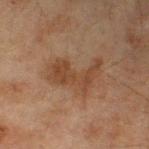| field | value |
|---|---|
| notes | imaged on a skin check; not biopsied |
| size | about 5.5 mm |
| image-analysis metrics | a footprint of about 11 mm² and two-axis asymmetry of about 0.5; a lesion color around L≈35 a*≈17 b*≈27 in CIELAB and roughly 7 lightness units darker than nearby skin; a border-irregularity index near 7/10, a within-lesion color-variation index near 2/10, and a peripheral color-asymmetry measure near 0.5; a classifier nevus-likeness of about 0/100 and lesion-presence confidence of about 100/100 |
| site | the left lower leg |
| acquisition | total-body-photography crop, ~15 mm field of view |
| tile lighting | cross-polarized illumination |
| patient | male, aged 43 to 47 |